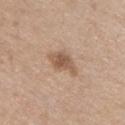Assessment: No biopsy was performed on this lesion — it was imaged during a full skin examination and was not determined to be concerning. Context: A male subject in their 60s. A close-up tile cropped from a whole-body skin photograph, about 15 mm across. Automated image analysis of the tile measured a lesion color around L≈55 a*≈18 b*≈30 in CIELAB, a lesion–skin lightness drop of about 11, and a normalized lesion–skin contrast near 7.5. The software also gave an automated nevus-likeness rating near 60 out of 100. The recorded lesion diameter is about 4 mm. The lesion is on the front of the torso.